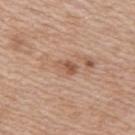Q: Was this lesion biopsied?
A: catalogued during a skin exam; not biopsied
Q: Lesion location?
A: the right upper arm
Q: Patient demographics?
A: female, aged approximately 40
Q: What is the imaging modality?
A: 15 mm crop, total-body photography